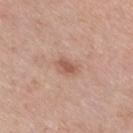Findings:
* workup · catalogued during a skin exam; not biopsied
* tile lighting · white-light
* image · ~15 mm tile from a whole-body skin photo
* site · the right thigh
* diameter · about 3 mm
* automated metrics · a lesion area of about 4.5 mm² and a shape eccentricity near 0.75; a mean CIELAB color near L≈57 a*≈22 b*≈28, about 10 CIELAB-L* units darker than the surrounding skin, and a normalized lesion–skin contrast near 6.5
* patient · male, aged 73 to 77